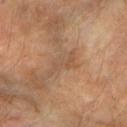Imaged during a routine full-body skin examination; the lesion was not biopsied and no histopathology is available.